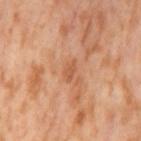Imaged during a routine full-body skin examination; the lesion was not biopsied and no histopathology is available. This is a cross-polarized tile. Measured at roughly 2.5 mm in maximum diameter. The patient is a female in their mid-50s. A 15 mm close-up extracted from a 3D total-body photography capture. The lesion is located on the left thigh.